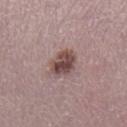Clinical impression: Part of a total-body skin-imaging series; this lesion was reviewed on a skin check and was not flagged for biopsy. Image and clinical context: From the right lower leg. Measured at roughly 3.5 mm in maximum diameter. An algorithmic analysis of the crop reported an average lesion color of about L≈46 a*≈19 b*≈19 (CIELAB). This image is a 15 mm lesion crop taken from a total-body photograph. Imaged with white-light lighting. The subject is a female aged 48 to 52.